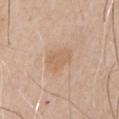Impression:
Recorded during total-body skin imaging; not selected for excision or biopsy.
Acquisition and patient details:
A close-up tile cropped from a whole-body skin photograph, about 15 mm across. On the chest. Approximately 4 mm at its widest. This is a white-light tile. A male subject, about 65 years old.